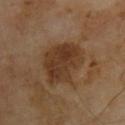{
  "biopsy_status": "not biopsied; imaged during a skin examination",
  "site": "upper back",
  "patient": {
    "sex": "male",
    "age_approx": 70
  },
  "image": {
    "source": "total-body photography crop",
    "field_of_view_mm": 15
  },
  "automated_metrics": {
    "area_mm2_approx": 20.0,
    "eccentricity": 0.7,
    "shape_asymmetry": 0.25,
    "cielab_L": 35,
    "cielab_a": 17,
    "cielab_b": 29,
    "vs_skin_darker_L": 9.0,
    "vs_skin_contrast_norm": 8.5,
    "border_irregularity_0_10": 3.0,
    "color_variation_0_10": 4.0,
    "peripheral_color_asymmetry": 1.5,
    "nevus_likeness_0_100": 15,
    "lesion_detection_confidence_0_100": 100
  }
}